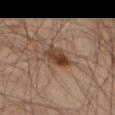workup: no biopsy performed (imaged during a skin exam)
subject: male, approximately 45 years of age
lesion diameter: ≈4 mm
anatomic site: the left thigh
automated metrics: a shape-asymmetry score of about 0.25 (0 = symmetric); a lesion color around L≈39 a*≈17 b*≈27 in CIELAB, a lesion–skin lightness drop of about 11, and a normalized border contrast of about 9.5; an automated nevus-likeness rating near 95 out of 100
image: ~15 mm tile from a whole-body skin photo